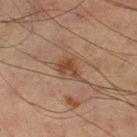{"biopsy_status": "not biopsied; imaged during a skin examination", "image": {"source": "total-body photography crop", "field_of_view_mm": 15}, "lesion_size": {"long_diameter_mm_approx": 3.0}, "site": "leg", "automated_metrics": {"peripheral_color_asymmetry": 1.0}, "lighting": "cross-polarized", "patient": {"sex": "male", "age_approx": 65}}